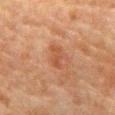notes = no biopsy performed (imaged during a skin exam); anatomic site = the abdomen; subject = male, approximately 80 years of age; acquisition = 15 mm crop, total-body photography; tile lighting = cross-polarized illumination; lesion size = ≈3 mm; automated lesion analysis = a classifier nevus-likeness of about 20/100 and lesion-presence confidence of about 100/100.